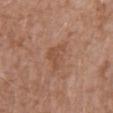Findings:
– workup — no biopsy performed (imaged during a skin exam)
– patient — male, in their 60s
– image — total-body-photography crop, ~15 mm field of view
– size — ~3.5 mm (longest diameter)
– tile lighting — white-light
– location — the mid back